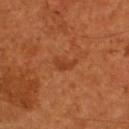<case>
<biopsy_status>not biopsied; imaged during a skin examination</biopsy_status>
<site>upper back</site>
<image>
  <source>total-body photography crop</source>
  <field_of_view_mm>15</field_of_view_mm>
</image>
<lesion_size>
  <long_diameter_mm_approx>3.0</long_diameter_mm_approx>
</lesion_size>
<patient>
  <sex>male</sex>
  <age_approx>55</age_approx>
</patient>
<lighting>cross-polarized</lighting>
</case>